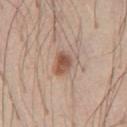Q: Was this lesion biopsied?
A: total-body-photography surveillance lesion; no biopsy
Q: What is the anatomic site?
A: the chest
Q: What lighting was used for the tile?
A: white-light
Q: Who is the patient?
A: male, about 45 years old
Q: What is the imaging modality?
A: ~15 mm tile from a whole-body skin photo
Q: How large is the lesion?
A: ~3 mm (longest diameter)